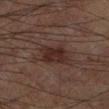{
  "biopsy_status": "not biopsied; imaged during a skin examination",
  "patient": {
    "sex": "male",
    "age_approx": 60
  },
  "image": {
    "source": "total-body photography crop",
    "field_of_view_mm": 15
  },
  "site": "leg"
}